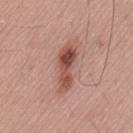Impression:
The lesion was tiled from a total-body skin photograph and was not biopsied.
Acquisition and patient details:
Imaged with white-light lighting. A lesion tile, about 15 mm wide, cut from a 3D total-body photograph. The subject is a male approximately 55 years of age. The lesion is on the lower back.